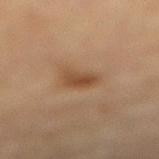follow-up = total-body-photography surveillance lesion; no biopsy
anatomic site = the right lower leg
image = 15 mm crop, total-body photography
patient = male, approximately 85 years of age or older